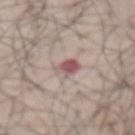<tbp_lesion>
<biopsy_status>not biopsied; imaged during a skin examination</biopsy_status>
<lesion_size>
  <long_diameter_mm_approx>2.5</long_diameter_mm_approx>
</lesion_size>
<automated_metrics>
  <area_mm2_approx>5.0</area_mm2_approx>
  <eccentricity>0.45</eccentricity>
  <shape_asymmetry>0.25</shape_asymmetry>
  <nevus_likeness_0_100>0</nevus_likeness_0_100>
  <lesion_detection_confidence_0_100>100</lesion_detection_confidence_0_100>
</automated_metrics>
<image>
  <source>total-body photography crop</source>
  <field_of_view_mm>15</field_of_view_mm>
</image>
<site>abdomen</site>
<patient>
  <sex>male</sex>
  <age_approx>65</age_approx>
</patient>
<lighting>white-light</lighting>
</tbp_lesion>